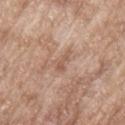The lesion was photographed on a routine skin check and not biopsied; there is no pathology result. Cropped from a total-body skin-imaging series; the visible field is about 15 mm. From the upper back. A male patient roughly 65 years of age.